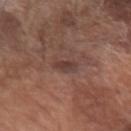The lesion was tiled from a total-body skin photograph and was not biopsied. A 15 mm close-up extracted from a 3D total-body photography capture. The subject is a male aged 68 to 72. The lesion is located on the left forearm. The lesion-visualizer software estimated a border-irregularity index near 3/10 and a color-variation rating of about 1.5/10. Captured under white-light illumination.